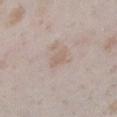Part of a total-body skin-imaging series; this lesion was reviewed on a skin check and was not flagged for biopsy. The lesion-visualizer software estimated a detector confidence of about 95 out of 100 that the crop contains a lesion. The subject is a female aged approximately 25. On the left lower leg. About 2.5 mm across. A close-up tile cropped from a whole-body skin photograph, about 15 mm across.